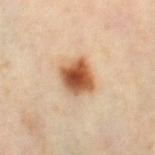follow-up: no biopsy performed (imaged during a skin exam)
TBP lesion metrics: an outline eccentricity of about 0.45 (0 = round, 1 = elongated); about 18 CIELAB-L* units darker than the surrounding skin and a normalized border contrast of about 12.5; a color-variation rating of about 7/10 and peripheral color asymmetry of about 2
lighting: cross-polarized illumination
lesion size: ≈3.5 mm
body site: the right thigh
image source: total-body-photography crop, ~15 mm field of view
patient: female, aged around 50